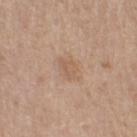Notes:
- workup: imaged on a skin check; not biopsied
- image: ~15 mm crop, total-body skin-cancer survey
- location: the left thigh
- subject: male, in their mid-70s